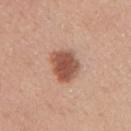follow-up — total-body-photography surveillance lesion; no biopsy | size — about 4 mm | site — the right upper arm | tile lighting — white-light illumination | automated lesion analysis — a lesion area of about 10 mm², an outline eccentricity of about 0.55 (0 = round, 1 = elongated), and a shape-asymmetry score of about 0.2 (0 = symmetric); an average lesion color of about L≈53 a*≈23 b*≈30 (CIELAB), about 15 CIELAB-L* units darker than the surrounding skin, and a normalized lesion–skin contrast near 10; a border-irregularity rating of about 2/10, a color-variation rating of about 3.5/10, and a peripheral color-asymmetry measure near 1 | subject — female, roughly 45 years of age | acquisition — 15 mm crop, total-body photography.